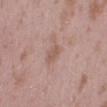Clinical impression:
No biopsy was performed on this lesion — it was imaged during a full skin examination and was not determined to be concerning.
Context:
The total-body-photography lesion software estimated an area of roughly 3.5 mm². The software also gave a nevus-likeness score of about 0/100 and lesion-presence confidence of about 100/100. Approximately 2.5 mm at its widest. A 15 mm crop from a total-body photograph taken for skin-cancer surveillance. A female subject, approximately 25 years of age. On the right lower leg. The tile uses white-light illumination.